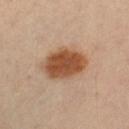Clinical impression:
Part of a total-body skin-imaging series; this lesion was reviewed on a skin check and was not flagged for biopsy.